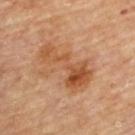Part of a total-body skin-imaging series; this lesion was reviewed on a skin check and was not flagged for biopsy. Cropped from a total-body skin-imaging series; the visible field is about 15 mm. The recorded lesion diameter is about 7 mm. A male patient, about 85 years old or older. An algorithmic analysis of the crop reported an automated nevus-likeness rating near 40 out of 100. Located on the upper back. Imaged with cross-polarized lighting.